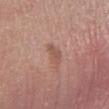  biopsy_status: not biopsied; imaged during a skin examination